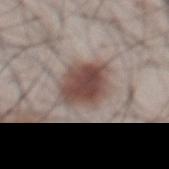Captured during whole-body skin photography for melanoma surveillance; the lesion was not biopsied.